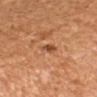The lesion was tiled from a total-body skin photograph and was not biopsied. The patient is a female aged 48 to 52. Located on the left forearm. A lesion tile, about 15 mm wide, cut from a 3D total-body photograph.